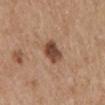Notes:
• size: ~3 mm (longest diameter)
• imaging modality: 15 mm crop, total-body photography
• patient: male, aged around 70
• site: the mid back
• TBP lesion metrics: an area of roughly 6.5 mm² and a shape eccentricity near 0.6; a lesion–skin lightness drop of about 15 and a normalized border contrast of about 10.5